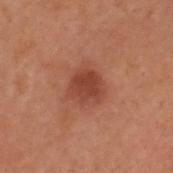| feature | finding |
|---|---|
| follow-up | no biopsy performed (imaged during a skin exam) |
| imaging modality | 15 mm crop, total-body photography |
| tile lighting | cross-polarized illumination |
| site | the head or neck |
| patient | male, aged around 50 |